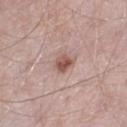Located on the left thigh. The subject is a male aged approximately 50. A 15 mm close-up tile from a total-body photography series done for melanoma screening. Approximately 2.5 mm at its widest.